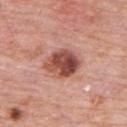follow-up — catalogued during a skin exam; not biopsied | imaging modality — ~15 mm tile from a whole-body skin photo | tile lighting — white-light illumination | patient — male, approximately 80 years of age | diameter — ≈4.5 mm | location — the upper back.